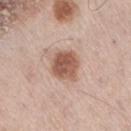No biopsy was performed on this lesion — it was imaged during a full skin examination and was not determined to be concerning. A 15 mm close-up tile from a total-body photography series done for melanoma screening. A male subject approximately 75 years of age. The lesion is on the right thigh. Approximately 3.5 mm at its widest.